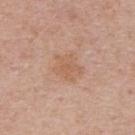No biopsy was performed on this lesion — it was imaged during a full skin examination and was not determined to be concerning. On the upper back. A 15 mm close-up tile from a total-body photography series done for melanoma screening. Captured under white-light illumination. A male subject aged around 65.